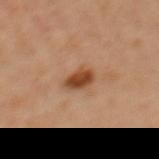Q: Is there a histopathology result?
A: imaged on a skin check; not biopsied
Q: What kind of image is this?
A: ~15 mm tile from a whole-body skin photo
Q: Where on the body is the lesion?
A: the back
Q: Who is the patient?
A: female, aged approximately 35
Q: What did automated image analysis measure?
A: border irregularity of about 2 on a 0–10 scale, internal color variation of about 3 on a 0–10 scale, and radial color variation of about 1; a nevus-likeness score of about 95/100 and a detector confidence of about 100 out of 100 that the crop contains a lesion
Q: Illumination type?
A: cross-polarized illumination
Q: How large is the lesion?
A: ~3 mm (longest diameter)